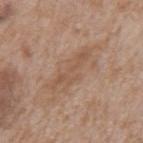Imaged during a routine full-body skin examination; the lesion was not biopsied and no histopathology is available. The subject is a male about 65 years old. Captured under white-light illumination. The lesion-visualizer software estimated a lesion color around L≈54 a*≈18 b*≈30 in CIELAB and about 7 CIELAB-L* units darker than the surrounding skin. And it measured a border-irregularity index near 9/10, internal color variation of about 1.5 on a 0–10 scale, and radial color variation of about 0.5. The software also gave a classifier nevus-likeness of about 0/100. The lesion is located on the mid back. The recorded lesion diameter is about 6 mm. A lesion tile, about 15 mm wide, cut from a 3D total-body photograph.